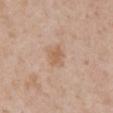{"biopsy_status": "not biopsied; imaged during a skin examination", "automated_metrics": {"nevus_likeness_0_100": 5, "lesion_detection_confidence_0_100": 100}, "patient": {"sex": "female", "age_approx": 30}, "site": "mid back", "image": {"source": "total-body photography crop", "field_of_view_mm": 15}, "lesion_size": {"long_diameter_mm_approx": 2.5}, "lighting": "white-light"}